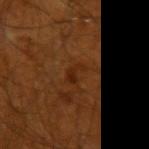Impression: No biopsy was performed on this lesion — it was imaged during a full skin examination and was not determined to be concerning. Clinical summary: Located on the left upper arm. Cropped from a whole-body photographic skin survey; the tile spans about 15 mm. Approximately 2.5 mm at its widest. This is a cross-polarized tile. A male subject about 70 years old.